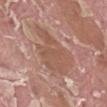The lesion was photographed on a routine skin check and not biopsied; there is no pathology result.